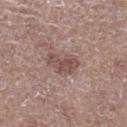  biopsy_status: not biopsied; imaged during a skin examination
  image:
    source: total-body photography crop
    field_of_view_mm: 15
  site: leg
  lighting: white-light
  patient:
    sex: male
    age_approx: 70
  automated_metrics:
    area_mm2_approx: 7.5
    eccentricity: 0.8
    shape_asymmetry: 0.35
    vs_skin_darker_L: 10.0